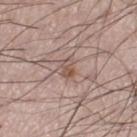Q: Was a biopsy performed?
A: imaged on a skin check; not biopsied
Q: Who is the patient?
A: male, aged 48 to 52
Q: How was the tile lit?
A: white-light
Q: What is the lesion's diameter?
A: ≈2.5 mm
Q: What kind of image is this?
A: total-body-photography crop, ~15 mm field of view
Q: What is the anatomic site?
A: the right thigh